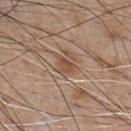Q: Was a biopsy performed?
A: no biopsy performed (imaged during a skin exam)
Q: What is the lesion's diameter?
A: ~3 mm (longest diameter)
Q: How was the tile lit?
A: white-light
Q: Patient demographics?
A: male, roughly 65 years of age
Q: What is the imaging modality?
A: 15 mm crop, total-body photography
Q: Lesion location?
A: the front of the torso